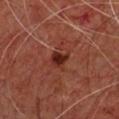This lesion was catalogued during total-body skin photography and was not selected for biopsy.
Automated tile analysis of the lesion measured a normalized border contrast of about 10. And it measured a nevus-likeness score of about 95/100 and lesion-presence confidence of about 100/100.
A male subject, about 60 years old.
Longest diameter approximately 2.5 mm.
The lesion is on the upper back.
Imaged with cross-polarized lighting.
A lesion tile, about 15 mm wide, cut from a 3D total-body photograph.